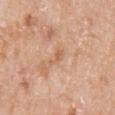Case summary:
* follow-up — imaged on a skin check; not biopsied
* patient — female, approximately 75 years of age
* illumination — white-light illumination
* body site — the right upper arm
* size — ~3 mm (longest diameter)
* image source — total-body-photography crop, ~15 mm field of view